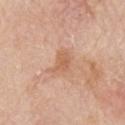– workup · catalogued during a skin exam; not biopsied
– image-analysis metrics · border irregularity of about 4.5 on a 0–10 scale, a within-lesion color-variation index near 2/10, and radial color variation of about 0.5
– imaging modality · ~15 mm tile from a whole-body skin photo
– patient · male, approximately 80 years of age
– size · ~3 mm (longest diameter)
– illumination · white-light illumination
– body site · the left arm